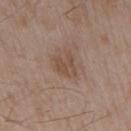Case summary:
– automated lesion analysis — a shape eccentricity near 0.65 and a symmetry-axis asymmetry near 0.3; an average lesion color of about L≈48 a*≈17 b*≈25 (CIELAB), roughly 7 lightness units darker than nearby skin, and a lesion-to-skin contrast of about 6 (normalized; higher = more distinct); a border-irregularity rating of about 4/10, a color-variation rating of about 2/10, and a peripheral color-asymmetry measure near 0.5; a nevus-likeness score of about 5/100
– subject — male, about 55 years old
– lighting — white-light illumination
– imaging modality — ~15 mm crop, total-body skin-cancer survey
– body site — the right upper arm